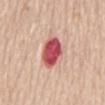Clinical impression: Recorded during total-body skin imaging; not selected for excision or biopsy. Image and clinical context: This is a white-light tile. On the mid back. A female patient aged 68–72. A region of skin cropped from a whole-body photographic capture, roughly 15 mm wide. Measured at roughly 4.5 mm in maximum diameter. The total-body-photography lesion software estimated a lesion area of about 10 mm², a shape eccentricity near 0.85, and two-axis asymmetry of about 0.15. It also reported a classifier nevus-likeness of about 0/100 and lesion-presence confidence of about 100/100.